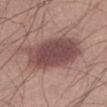Findings:
* follow-up · total-body-photography surveillance lesion; no biopsy